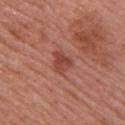<tbp_lesion>
<biopsy_status>not biopsied; imaged during a skin examination</biopsy_status>
<patient>
  <sex>female</sex>
  <age_approx>55</age_approx>
</patient>
<lighting>white-light</lighting>
<site>upper back</site>
<lesion_size>
  <long_diameter_mm_approx>3.0</long_diameter_mm_approx>
</lesion_size>
<image>
  <source>total-body photography crop</source>
  <field_of_view_mm>15</field_of_view_mm>
</image>
<automated_metrics>
  <area_mm2_approx>5.5</area_mm2_approx>
  <eccentricity>0.55</eccentricity>
  <shape_asymmetry>0.4</shape_asymmetry>
  <border_irregularity_0_10>3.5</border_irregularity_0_10>
  <color_variation_0_10>3.0</color_variation_0_10>
  <peripheral_color_asymmetry>1.0</peripheral_color_asymmetry>
</automated_metrics>
</tbp_lesion>